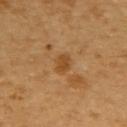Imaged during a routine full-body skin examination; the lesion was not biopsied and no histopathology is available.
A female subject, aged 53–57.
The lesion's longest dimension is about 2.5 mm.
The tile uses cross-polarized illumination.
Automated image analysis of the tile measured a lesion color around L≈49 a*≈20 b*≈41 in CIELAB and roughly 7 lightness units darker than nearby skin. It also reported a border-irregularity rating of about 2/10, a color-variation rating of about 2.5/10, and peripheral color asymmetry of about 1. It also reported a lesion-detection confidence of about 100/100.
On the upper back.
A close-up tile cropped from a whole-body skin photograph, about 15 mm across.